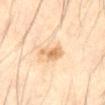<record>
<biopsy_status>not biopsied; imaged during a skin examination</biopsy_status>
<site>abdomen</site>
<patient>
  <sex>male</sex>
  <age_approx>60</age_approx>
</patient>
<image>
  <source>total-body photography crop</source>
  <field_of_view_mm>15</field_of_view_mm>
</image>
</record>